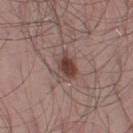| key | value |
|---|---|
| biopsy status | total-body-photography surveillance lesion; no biopsy |
| site | the left thigh |
| TBP lesion metrics | a mean CIELAB color near L≈43 a*≈19 b*≈22 and a lesion-to-skin contrast of about 9 (normalized; higher = more distinct); radial color variation of about 1; a nevus-likeness score of about 95/100 and lesion-presence confidence of about 100/100 |
| lesion diameter | ≈4 mm |
| imaging modality | ~15 mm tile from a whole-body skin photo |
| lighting | white-light illumination |
| patient | male, roughly 55 years of age |